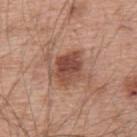Recorded during total-body skin imaging; not selected for excision or biopsy. The patient is a male aged 53–57. The lesion is on the upper back. This image is a 15 mm lesion crop taken from a total-body photograph. Automated image analysis of the tile measured a footprint of about 10 mm², an outline eccentricity of about 0.6 (0 = round, 1 = elongated), and a symmetry-axis asymmetry near 0.2. The analysis additionally found an average lesion color of about L≈48 a*≈23 b*≈28 (CIELAB), roughly 12 lightness units darker than nearby skin, and a normalized lesion–skin contrast near 8.5. The software also gave lesion-presence confidence of about 100/100. Longest diameter approximately 4 mm. Imaged with white-light lighting.